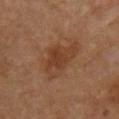notes=imaged on a skin check; not biopsied | lesion size=~6 mm (longest diameter) | image=15 mm crop, total-body photography | anatomic site=the chest | illumination=cross-polarized | subject=male, about 55 years old.